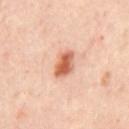Q: Is there a histopathology result?
A: no biopsy performed (imaged during a skin exam)
Q: Lesion size?
A: about 3.5 mm
Q: How was the tile lit?
A: cross-polarized
Q: What is the anatomic site?
A: the chest
Q: How was this image acquired?
A: 15 mm crop, total-body photography
Q: What are the patient's age and sex?
A: aged 53–57